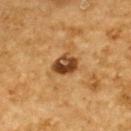Impression:
Captured during whole-body skin photography for melanoma surveillance; the lesion was not biopsied.
Context:
The lesion is on the upper back. A region of skin cropped from a whole-body photographic capture, roughly 15 mm wide. Measured at roughly 3.5 mm in maximum diameter. A male subject aged around 85 or older. The tile uses cross-polarized illumination.